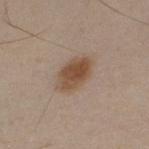This lesion was catalogued during total-body skin photography and was not selected for biopsy. A male patient, approximately 30 years of age. Longest diameter approximately 4.5 mm. Automated tile analysis of the lesion measured a lesion area of about 10 mm², an eccentricity of roughly 0.8, and two-axis asymmetry of about 0.15. And it measured internal color variation of about 4.5 on a 0–10 scale and radial color variation of about 1.5. The software also gave a classifier nevus-likeness of about 100/100. This is a white-light tile. The lesion is on the upper back. A 15 mm crop from a total-body photograph taken for skin-cancer surveillance.